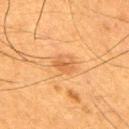Recorded during total-body skin imaging; not selected for excision or biopsy.
Located on the right upper arm.
The recorded lesion diameter is about 3 mm.
A close-up tile cropped from a whole-body skin photograph, about 15 mm across.
Captured under cross-polarized illumination.
A male patient aged 48–52.